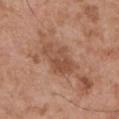Assessment:
Imaged during a routine full-body skin examination; the lesion was not biopsied and no histopathology is available.
Clinical summary:
Approximately 5 mm at its widest. A roughly 15 mm field-of-view crop from a total-body skin photograph. A male subject roughly 55 years of age. From the left upper arm. Imaged with white-light lighting.